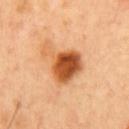{"lighting": "cross-polarized", "site": "chest", "automated_metrics": {"eccentricity": 0.6, "shape_asymmetry": 0.5, "border_irregularity_0_10": 5.5, "color_variation_0_10": 7.0, "peripheral_color_asymmetry": 2.0}, "image": {"source": "total-body photography crop", "field_of_view_mm": 15}, "patient": {"sex": "male", "age_approx": 50}, "lesion_size": {"long_diameter_mm_approx": 5.5}}